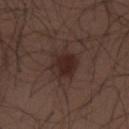Impression:
Imaged during a routine full-body skin examination; the lesion was not biopsied and no histopathology is available.
Image and clinical context:
A male subject, in their 30s. From the upper back. Measured at roughly 3.5 mm in maximum diameter. A lesion tile, about 15 mm wide, cut from a 3D total-body photograph.